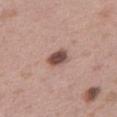biopsy status: imaged on a skin check; not biopsied | lesion size: about 3 mm | patient: female, in their 40s | acquisition: ~15 mm tile from a whole-body skin photo | site: the abdomen | illumination: white-light.